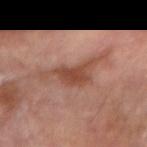Imaged during a routine full-body skin examination; the lesion was not biopsied and no histopathology is available. Cropped from a total-body skin-imaging series; the visible field is about 15 mm. A male patient roughly 65 years of age. On the right forearm.